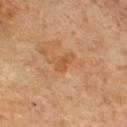follow-up: no biopsy performed (imaged during a skin exam) | image source: ~15 mm crop, total-body skin-cancer survey | patient: male, aged 58–62 | illumination: cross-polarized | diameter: about 2.5 mm | anatomic site: the chest.